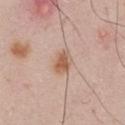biopsy_status: not biopsied; imaged during a skin examination
patient:
  sex: male
  age_approx: 60
image:
  source: total-body photography crop
  field_of_view_mm: 15
automated_metrics:
  cielab_L: 59
  cielab_a: 20
  cielab_b: 31
  border_irregularity_0_10: 1.5
  color_variation_0_10: 5.0
  peripheral_color_asymmetry: 1.5
  nevus_likeness_0_100: 85
  lesion_detection_confidence_0_100: 100
site: chest
lighting: white-light
lesion_size:
  long_diameter_mm_approx: 3.0